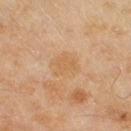  biopsy_status: not biopsied; imaged during a skin examination
  lesion_size:
    long_diameter_mm_approx: 3.5
  patient:
    sex: female
    age_approx: 60
  site: leg
  lighting: cross-polarized
  image:
    source: total-body photography crop
    field_of_view_mm: 15